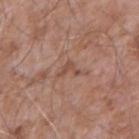follow-up: imaged on a skin check; not biopsied
subject: male, in their mid- to late 70s
body site: the right upper arm
diameter: ~2.5 mm (longest diameter)
image source: ~15 mm tile from a whole-body skin photo
automated metrics: a mean CIELAB color near L≈49 a*≈21 b*≈27, about 8 CIELAB-L* units darker than the surrounding skin, and a lesion-to-skin contrast of about 6 (normalized; higher = more distinct); border irregularity of about 7 on a 0–10 scale and a color-variation rating of about 0/10